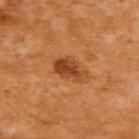Recorded during total-body skin imaging; not selected for excision or biopsy. A female patient, aged around 55. This is a cross-polarized tile. On the upper back. The recorded lesion diameter is about 4 mm. A lesion tile, about 15 mm wide, cut from a 3D total-body photograph.